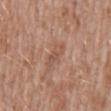diameter = ~3 mm (longest diameter) | image = total-body-photography crop, ~15 mm field of view | automated lesion analysis = a lesion color around L≈53 a*≈21 b*≈28 in CIELAB; border irregularity of about 3 on a 0–10 scale, a within-lesion color-variation index near 2.5/10, and a peripheral color-asymmetry measure near 0.5; a detector confidence of about 100 out of 100 that the crop contains a lesion | subject = male, aged 58–62 | lighting = white-light | site = the front of the torso.